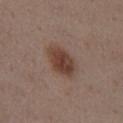notes = catalogued during a skin exam; not biopsied
image-analysis metrics = a lesion color around L≈40 a*≈18 b*≈25 in CIELAB and a normalized lesion–skin contrast near 9.5; a border-irregularity rating of about 1.5/10 and internal color variation of about 4 on a 0–10 scale; lesion-presence confidence of about 100/100
tile lighting = white-light illumination
acquisition = ~15 mm tile from a whole-body skin photo
lesion diameter = ~4 mm (longest diameter)
patient = male, approximately 55 years of age
location = the front of the torso